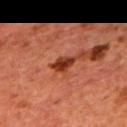Case summary:
- biopsy status: no biopsy performed (imaged during a skin exam)
- lesion diameter: ~3 mm (longest diameter)
- anatomic site: the back
- acquisition: 15 mm crop, total-body photography
- automated metrics: a lesion area of about 4 mm², an eccentricity of roughly 0.85, and a shape-asymmetry score of about 0.2 (0 = symmetric); a mean CIELAB color near L≈33 a*≈30 b*≈32 and a normalized lesion–skin contrast near 11.5; peripheral color asymmetry of about 1
- patient: male, aged 63 to 67